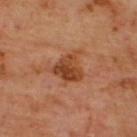follow-up: no biopsy performed (imaged during a skin exam) | TBP lesion metrics: a lesion area of about 9 mm² and an outline eccentricity of about 0.4 (0 = round, 1 = elongated); border irregularity of about 4 on a 0–10 scale, internal color variation of about 5.5 on a 0–10 scale, and radial color variation of about 2 | tile lighting: cross-polarized | location: the upper back | imaging modality: 15 mm crop, total-body photography | lesion diameter: ≈4 mm | patient: male, aged around 65.